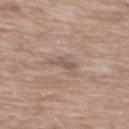Impression:
The lesion was photographed on a routine skin check and not biopsied; there is no pathology result.
Acquisition and patient details:
A male subject, in their 60s. On the chest. A 15 mm close-up tile from a total-body photography series done for melanoma screening.